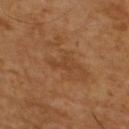{"biopsy_status": "not biopsied; imaged during a skin examination", "site": "upper back", "patient": {"sex": "male", "age_approx": 65}, "image": {"source": "total-body photography crop", "field_of_view_mm": 15}, "lighting": "cross-polarized", "lesion_size": {"long_diameter_mm_approx": 3.5}, "automated_metrics": {"cielab_L": 40, "cielab_a": 21, "cielab_b": 33, "vs_skin_contrast_norm": 5.0, "color_variation_0_10": 1.0, "peripheral_color_asymmetry": 0.5}}